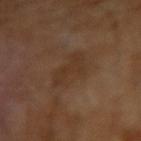{
  "biopsy_status": "not biopsied; imaged during a skin examination",
  "lighting": "cross-polarized",
  "image": {
    "source": "total-body photography crop",
    "field_of_view_mm": 15
  },
  "patient": {
    "sex": "male",
    "age_approx": 65
  }
}